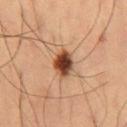* follow-up — catalogued during a skin exam; not biopsied
* subject — male, about 60 years old
* body site — the right thigh
* lighting — cross-polarized
* size — ~3.5 mm (longest diameter)
* acquisition — ~15 mm crop, total-body skin-cancer survey